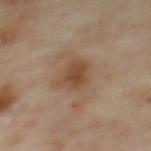Assessment: No biopsy was performed on this lesion — it was imaged during a full skin examination and was not determined to be concerning. Background: The lesion is on the upper back. Measured at roughly 3.5 mm in maximum diameter. A female subject about 60 years old. Captured under cross-polarized illumination. An algorithmic analysis of the crop reported a border-irregularity rating of about 2.5/10, a within-lesion color-variation index near 3.5/10, and radial color variation of about 1. The analysis additionally found an automated nevus-likeness rating near 50 out of 100. A roughly 15 mm field-of-view crop from a total-body skin photograph.